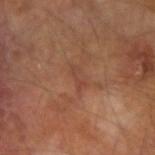A close-up tile cropped from a whole-body skin photograph, about 15 mm across.
Automated image analysis of the tile measured a footprint of about 2.5 mm², an outline eccentricity of about 0.95 (0 = round, 1 = elongated), and a symmetry-axis asymmetry near 0.45. It also reported a within-lesion color-variation index near 0/10 and radial color variation of about 0.
The subject is a male aged around 65.
On the left arm.